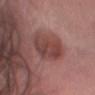Imaged during a routine full-body skin examination; the lesion was not biopsied and no histopathology is available. Measured at roughly 4.5 mm in maximum diameter. A 15 mm close-up extracted from a 3D total-body photography capture. Located on the head or neck. The subject is a male aged approximately 50. Imaged with white-light lighting. The total-body-photography lesion software estimated a within-lesion color-variation index near 5/10 and radial color variation of about 1.5. The software also gave an automated nevus-likeness rating near 50 out of 100 and lesion-presence confidence of about 100/100.